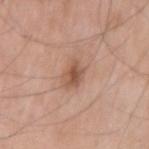workup = catalogued during a skin exam; not biopsied
lighting = white-light illumination
size = ~2.5 mm (longest diameter)
site = the right upper arm
automated metrics = a mean CIELAB color near L≈53 a*≈21 b*≈30 and a normalized lesion–skin contrast near 7.5; an automated nevus-likeness rating near 60 out of 100 and a lesion-detection confidence of about 100/100
patient = male, aged 73–77
image = total-body-photography crop, ~15 mm field of view